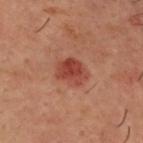follow-up=total-body-photography surveillance lesion; no biopsy
illumination=cross-polarized
subject=male, in their 50s
image=~15 mm crop, total-body skin-cancer survey
size=≈3.5 mm
image-analysis metrics=a nevus-likeness score of about 95/100 and lesion-presence confidence of about 100/100
location=the head or neck